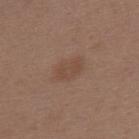<case>
  <biopsy_status>not biopsied; imaged during a skin examination</biopsy_status>
  <patient>
    <sex>female</sex>
    <age_approx>45</age_approx>
  </patient>
  <lesion_size>
    <long_diameter_mm_approx>4.0</long_diameter_mm_approx>
  </lesion_size>
  <site>upper back</site>
  <automated_metrics>
    <cielab_L>46</cielab_L>
    <cielab_a>17</cielab_a>
    <cielab_b>27</cielab_b>
    <vs_skin_contrast_norm>5.0</vs_skin_contrast_norm>
    <nevus_likeness_0_100>15</nevus_likeness_0_100>
    <lesion_detection_confidence_0_100>100</lesion_detection_confidence_0_100>
  </automated_metrics>
  <image>
    <source>total-body photography crop</source>
    <field_of_view_mm>15</field_of_view_mm>
  </image>
</case>